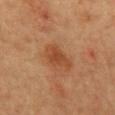Findings:
- workup: total-body-photography surveillance lesion; no biopsy
- tile lighting: cross-polarized illumination
- TBP lesion metrics: a mean CIELAB color near L≈41 a*≈22 b*≈33, about 8 CIELAB-L* units darker than the surrounding skin, and a lesion-to-skin contrast of about 7 (normalized; higher = more distinct)
- site: the mid back
- image: ~15 mm crop, total-body skin-cancer survey
- subject: female, aged 58 to 62
- size: ≈4.5 mm